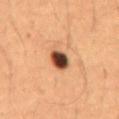Imaged during a routine full-body skin examination; the lesion was not biopsied and no histopathology is available. The lesion is on the front of the torso. The patient is a male about 50 years old. A lesion tile, about 15 mm wide, cut from a 3D total-body photograph. Approximately 2.5 mm at its widest. The tile uses cross-polarized illumination.